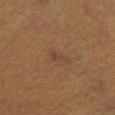Captured during whole-body skin photography for melanoma surveillance; the lesion was not biopsied.
The lesion-visualizer software estimated a lesion area of about 2.5 mm², an eccentricity of roughly 0.95, and a shape-asymmetry score of about 0.5 (0 = symmetric). The software also gave a mean CIELAB color near L≈32 a*≈14 b*≈22 and roughly 4 lightness units darker than nearby skin.
A roughly 15 mm field-of-view crop from a total-body skin photograph.
The lesion is on the left lower leg.
Captured under cross-polarized illumination.
About 3 mm across.
A male patient aged around 50.